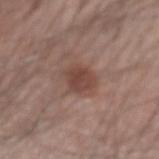Clinical impression:
No biopsy was performed on this lesion — it was imaged during a full skin examination and was not determined to be concerning.
Image and clinical context:
On the left forearm. A 15 mm close-up tile from a total-body photography series done for melanoma screening. The tile uses white-light illumination. Automated tile analysis of the lesion measured border irregularity of about 1.5 on a 0–10 scale and a within-lesion color-variation index near 2.5/10. And it measured a classifier nevus-likeness of about 85/100 and a lesion-detection confidence of about 100/100. A male patient, in their mid-40s.